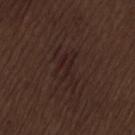Q: Is there a histopathology result?
A: no biopsy performed (imaged during a skin exam)
Q: How was the tile lit?
A: white-light
Q: Lesion location?
A: the lower back
Q: Who is the patient?
A: male, aged 68 to 72
Q: Lesion size?
A: about 4 mm
Q: How was this image acquired?
A: ~15 mm crop, total-body skin-cancer survey